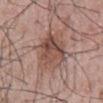location — the mid back; patient — male, aged 53 to 57; acquisition — ~15 mm tile from a whole-body skin photo; lesion size — about 7 mm.